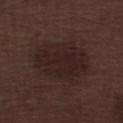workup = total-body-photography surveillance lesion; no biopsy
lighting = white-light
acquisition = 15 mm crop, total-body photography
lesion diameter = about 6.5 mm
TBP lesion metrics = an area of roughly 25 mm², an eccentricity of roughly 0.7, and two-axis asymmetry of about 0.15; border irregularity of about 2 on a 0–10 scale and a peripheral color-asymmetry measure near 1; a lesion-detection confidence of about 100/100
patient = male, about 70 years old
anatomic site = the left lower leg